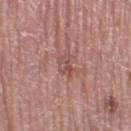{"biopsy_status": "not biopsied; imaged during a skin examination", "patient": {"sex": "female", "age_approx": 65}, "image": {"source": "total-body photography crop", "field_of_view_mm": 15}, "site": "right thigh", "lighting": "white-light", "automated_metrics": {"cielab_L": 51, "cielab_a": 22, "cielab_b": 24, "vs_skin_contrast_norm": 5.5, "border_irregularity_0_10": 2.5, "nevus_likeness_0_100": 0, "lesion_detection_confidence_0_100": 90}, "lesion_size": {"long_diameter_mm_approx": 3.0}}